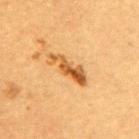Notes:
* illumination — cross-polarized illumination
* automated lesion analysis — an average lesion color of about L≈50 a*≈23 b*≈41 (CIELAB), a lesion–skin lightness drop of about 13, and a normalized lesion–skin contrast near 9.5; border irregularity of about 5.5 on a 0–10 scale and peripheral color asymmetry of about 2
* location — the mid back
* acquisition — total-body-photography crop, ~15 mm field of view
* patient — female, aged around 55